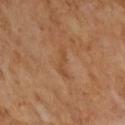Assessment:
Recorded during total-body skin imaging; not selected for excision or biopsy.
Acquisition and patient details:
A male patient aged approximately 65. A 15 mm close-up extracted from a 3D total-body photography capture. Imaged with cross-polarized lighting. The lesion-visualizer software estimated a footprint of about 3.5 mm², an outline eccentricity of about 0.95 (0 = round, 1 = elongated), and a symmetry-axis asymmetry near 0.4. And it measured a lesion-to-skin contrast of about 5 (normalized; higher = more distinct). The analysis additionally found border irregularity of about 5.5 on a 0–10 scale, a within-lesion color-variation index near 0/10, and radial color variation of about 0. And it measured an automated nevus-likeness rating near 0 out of 100.